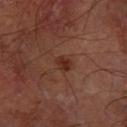– follow-up — catalogued during a skin exam; not biopsied
– location — the left forearm
– image source — ~15 mm crop, total-body skin-cancer survey
– subject — male, aged 63–67